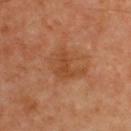Captured during whole-body skin photography for melanoma surveillance; the lesion was not biopsied. A close-up tile cropped from a whole-body skin photograph, about 15 mm across. The subject is a male in their 50s. Located on the upper back.